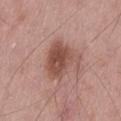The lesion was photographed on a routine skin check and not biopsied; there is no pathology result.